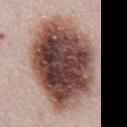The lesion was tiled from a total-body skin photograph and was not biopsied. The lesion-visualizer software estimated a footprint of about 70 mm² and an eccentricity of roughly 0.8. The software also gave a lesion color around L≈47 a*≈19 b*≈22 in CIELAB, about 23 CIELAB-L* units darker than the surrounding skin, and a normalized lesion–skin contrast near 15. It also reported an automated nevus-likeness rating near 100 out of 100 and a lesion-detection confidence of about 100/100. Cropped from a whole-body photographic skin survey; the tile spans about 15 mm. The patient is a female about 50 years old. Imaged with white-light lighting. Measured at roughly 12.5 mm in maximum diameter. Located on the chest.